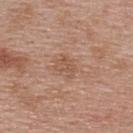<lesion>
  <biopsy_status>not biopsied; imaged during a skin examination</biopsy_status>
  <lesion_size>
    <long_diameter_mm_approx>2.5</long_diameter_mm_approx>
  </lesion_size>
  <image>
    <source>total-body photography crop</source>
    <field_of_view_mm>15</field_of_view_mm>
  </image>
  <lighting>white-light</lighting>
  <site>upper back</site>
  <patient>
    <sex>female</sex>
    <age_approx>40</age_approx>
  </patient>
  <automated_metrics>
    <cielab_L>54</cielab_L>
    <cielab_a>20</cielab_a>
    <cielab_b>30</cielab_b>
    <vs_skin_darker_L>7.0</vs_skin_darker_L>
    <vs_skin_contrast_norm>5.5</vs_skin_contrast_norm>
    <border_irregularity_0_10>4.5</border_irregularity_0_10>
    <color_variation_0_10>1.5</color_variation_0_10>
    <peripheral_color_asymmetry>0.5</peripheral_color_asymmetry>
  </automated_metrics>
</lesion>